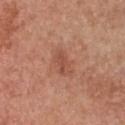notes: imaged on a skin check; not biopsied
automated metrics: a normalized border contrast of about 6; internal color variation of about 0.5 on a 0–10 scale and peripheral color asymmetry of about 0
diameter: ~2.5 mm (longest diameter)
lighting: white-light
acquisition: ~15 mm tile from a whole-body skin photo
patient: female, aged approximately 50
anatomic site: the front of the torso